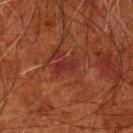Notes:
- workup · imaged on a skin check; not biopsied
- acquisition · ~15 mm tile from a whole-body skin photo
- body site · the left forearm
- lighting · cross-polarized
- patient · male, in their 80s